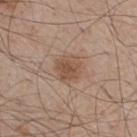The lesion was photographed on a routine skin check and not biopsied; there is no pathology result. A 15 mm crop from a total-body photograph taken for skin-cancer surveillance. The lesion's longest dimension is about 3 mm. An algorithmic analysis of the crop reported a border-irregularity index near 1.5/10, a within-lesion color-variation index near 3/10, and a peripheral color-asymmetry measure near 1. It also reported an automated nevus-likeness rating near 55 out of 100. On the leg. The subject is a male in their mid-40s.